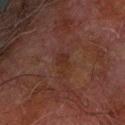notes = imaged on a skin check; not biopsied
subject = male, approximately 75 years of age
lesion diameter = ~3 mm (longest diameter)
imaging modality = ~15 mm crop, total-body skin-cancer survey
lighting = cross-polarized
location = the right forearm